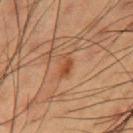No biopsy was performed on this lesion — it was imaged during a full skin examination and was not determined to be concerning.
Located on the arm.
A region of skin cropped from a whole-body photographic capture, roughly 15 mm wide.
The patient is a male in their mid- to late 50s.